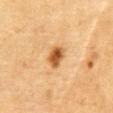This lesion was catalogued during total-body skin photography and was not selected for biopsy.
A roughly 15 mm field-of-view crop from a total-body skin photograph.
From the front of the torso.
Automated tile analysis of the lesion measured a mean CIELAB color near L≈49 a*≈21 b*≈38, about 14 CIELAB-L* units darker than the surrounding skin, and a normalized border contrast of about 10. The software also gave a border-irregularity index near 2/10, a within-lesion color-variation index near 4.5/10, and radial color variation of about 1.5.
This is a cross-polarized tile.
A male patient, aged 83 or over.
Longest diameter approximately 3 mm.